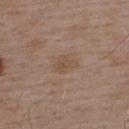<tbp_lesion>
  <biopsy_status>not biopsied; imaged during a skin examination</biopsy_status>
  <site>upper back</site>
  <image>
    <source>total-body photography crop</source>
    <field_of_view_mm>15</field_of_view_mm>
  </image>
  <lighting>white-light</lighting>
  <automated_metrics>
    <cielab_L>50</cielab_L>
    <cielab_a>16</cielab_a>
    <cielab_b>29</cielab_b>
    <vs_skin_darker_L>6.0</vs_skin_darker_L>
    <vs_skin_contrast_norm>5.5</vs_skin_contrast_norm>
    <border_irregularity_0_10>3.0</border_irregularity_0_10>
    <peripheral_color_asymmetry>1.0</peripheral_color_asymmetry>
  </automated_metrics>
  <patient>
    <sex>male</sex>
    <age_approx>55</age_approx>
  </patient>
</tbp_lesion>